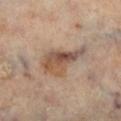Imaged during a routine full-body skin examination; the lesion was not biopsied and no histopathology is available.
A female subject roughly 60 years of age.
The total-body-photography lesion software estimated an area of roughly 12 mm² and a shape eccentricity near 0.85.
The recorded lesion diameter is about 6 mm.
This image is a 15 mm lesion crop taken from a total-body photograph.
From the right lower leg.
Captured under cross-polarized illumination.